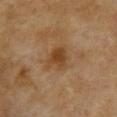The lesion was tiled from a total-body skin photograph and was not biopsied.
This image is a 15 mm lesion crop taken from a total-body photograph.
The lesion is on the front of the torso.
A female subject, roughly 60 years of age.
Measured at roughly 3.5 mm in maximum diameter.
The tile uses cross-polarized illumination.
Automated tile analysis of the lesion measured a lesion color around L≈39 a*≈17 b*≈33 in CIELAB, a lesion–skin lightness drop of about 8, and a normalized lesion–skin contrast near 7.5. The software also gave a nevus-likeness score of about 45/100.